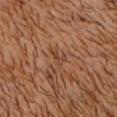{
  "biopsy_status": "not biopsied; imaged during a skin examination",
  "lesion_size": {
    "long_diameter_mm_approx": 2.5
  },
  "image": {
    "source": "total-body photography crop",
    "field_of_view_mm": 15
  },
  "site": "front of the torso",
  "lighting": "cross-polarized",
  "patient": {
    "sex": "male",
    "age_approx": 30
  },
  "automated_metrics": {
    "area_mm2_approx": 2.5,
    "eccentricity": 0.8,
    "color_variation_0_10": 0.0,
    "peripheral_color_asymmetry": 0.0
  }
}